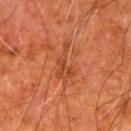Part of a total-body skin-imaging series; this lesion was reviewed on a skin check and was not flagged for biopsy. A region of skin cropped from a whole-body photographic capture, roughly 15 mm wide. The lesion is on the left upper arm. Imaged with cross-polarized lighting. Measured at roughly 4 mm in maximum diameter. The patient is a male aged approximately 80.